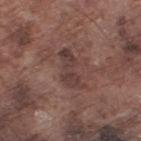notes = imaged on a skin check; not biopsied
patient = male, in their mid-70s
illumination = white-light
body site = the right lower leg
image = 15 mm crop, total-body photography